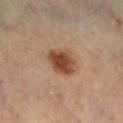follow-up = imaged on a skin check; not biopsied
site = the right lower leg
automated metrics = a border-irregularity rating of about 1.5/10, internal color variation of about 4 on a 0–10 scale, and radial color variation of about 1
patient = female, roughly 60 years of age
illumination = cross-polarized
imaging modality = ~15 mm tile from a whole-body skin photo